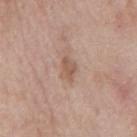<case>
  <patient>
    <sex>male</sex>
    <age_approx>60</age_approx>
  </patient>
  <site>mid back</site>
  <image>
    <source>total-body photography crop</source>
    <field_of_view_mm>15</field_of_view_mm>
  </image>
  <lesion_size>
    <long_diameter_mm_approx>2.5</long_diameter_mm_approx>
  </lesion_size>
</case>